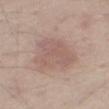Impression:
Imaged during a routine full-body skin examination; the lesion was not biopsied and no histopathology is available.
Clinical summary:
Cropped from a whole-body photographic skin survey; the tile spans about 15 mm. The lesion's longest dimension is about 5 mm. The tile uses white-light illumination. A male patient approximately 65 years of age. From the right thigh.